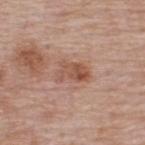{
  "biopsy_status": "not biopsied; imaged during a skin examination",
  "site": "upper back",
  "lighting": "white-light",
  "automated_metrics": {
    "eccentricity": 0.8,
    "shape_asymmetry": 0.25,
    "lesion_detection_confidence_0_100": 100
  },
  "lesion_size": {
    "long_diameter_mm_approx": 3.5
  },
  "patient": {
    "sex": "male",
    "age_approx": 65
  },
  "image": {
    "source": "total-body photography crop",
    "field_of_view_mm": 15
  }
}